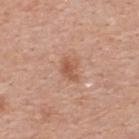<case>
  <biopsy_status>not biopsied; imaged during a skin examination</biopsy_status>
  <site>back</site>
  <image>
    <source>total-body photography crop</source>
    <field_of_view_mm>15</field_of_view_mm>
  </image>
  <patient>
    <sex>female</sex>
    <age_approx>40</age_approx>
  </patient>
</case>